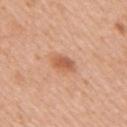Impression:
The lesion was tiled from a total-body skin photograph and was not biopsied.
Context:
A female subject, aged approximately 40. On the right upper arm. The lesion-visualizer software estimated a border-irregularity index near 2/10 and a color-variation rating of about 2.5/10. Cropped from a whole-body photographic skin survey; the tile spans about 15 mm. The tile uses white-light illumination. Longest diameter approximately 3 mm.